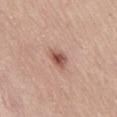Recorded during total-body skin imaging; not selected for excision or biopsy. This is a white-light tile. A male patient, about 65 years old. About 3 mm across. The lesion-visualizer software estimated a footprint of about 4.5 mm² and a shape eccentricity near 0.6. The software also gave a mean CIELAB color near L≈54 a*≈23 b*≈27, about 13 CIELAB-L* units darker than the surrounding skin, and a normalized border contrast of about 9. The analysis additionally found border irregularity of about 2.5 on a 0–10 scale, internal color variation of about 4.5 on a 0–10 scale, and a peripheral color-asymmetry measure near 1.5. The lesion is on the abdomen. A lesion tile, about 15 mm wide, cut from a 3D total-body photograph.